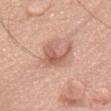Recorded during total-body skin imaging; not selected for excision or biopsy.
From the chest.
A male subject, in their mid- to late 70s.
The recorded lesion diameter is about 3.5 mm.
A 15 mm close-up tile from a total-body photography series done for melanoma screening.